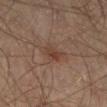Impression: No biopsy was performed on this lesion — it was imaged during a full skin examination and was not determined to be concerning. Image and clinical context: Imaged with cross-polarized lighting. Located on the left thigh. Automated image analysis of the tile measured a mean CIELAB color near L≈39 a*≈18 b*≈26 and roughly 7 lightness units darker than nearby skin. The software also gave border irregularity of about 3 on a 0–10 scale and a peripheral color-asymmetry measure near 1.5. And it measured lesion-presence confidence of about 100/100. A region of skin cropped from a whole-body photographic capture, roughly 15 mm wide. A male subject roughly 65 years of age. Longest diameter approximately 2.5 mm.